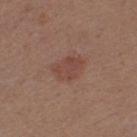Assessment:
The lesion was tiled from a total-body skin photograph and was not biopsied.
Context:
The recorded lesion diameter is about 4 mm. Located on the left thigh. Captured under white-light illumination. The lesion-visualizer software estimated a border-irregularity index near 2/10, a within-lesion color-variation index near 2.5/10, and radial color variation of about 1. It also reported a nevus-likeness score of about 30/100 and lesion-presence confidence of about 100/100. The subject is a female approximately 30 years of age. This image is a 15 mm lesion crop taken from a total-body photograph.